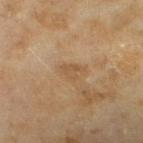Notes:
* illumination: cross-polarized illumination
* location: the left lower leg
* automated lesion analysis: an average lesion color of about L≈48 a*≈15 b*≈33 (CIELAB) and a normalized border contrast of about 5; a peripheral color-asymmetry measure near 0.5
* size: about 3 mm
* image: ~15 mm crop, total-body skin-cancer survey
* patient: female, aged approximately 60